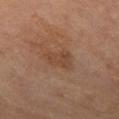{
  "biopsy_status": "not biopsied; imaged during a skin examination"
}